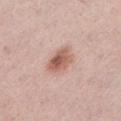Notes:
- workup — no biopsy performed (imaged during a skin exam)
- imaging modality — ~15 mm crop, total-body skin-cancer survey
- site — the left lower leg
- subject — female, aged around 45
- lesion diameter — about 4 mm
- automated lesion analysis — a lesion color around L≈59 a*≈22 b*≈27 in CIELAB, about 12 CIELAB-L* units darker than the surrounding skin, and a normalized border contrast of about 8; a border-irregularity rating of about 2/10, a within-lesion color-variation index near 5/10, and radial color variation of about 2; an automated nevus-likeness rating near 85 out of 100
- illumination — white-light